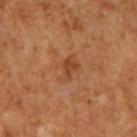Imaged during a routine full-body skin examination; the lesion was not biopsied and no histopathology is available. A male patient in their mid-60s. Imaged with cross-polarized lighting. A 15 mm crop from a total-body photograph taken for skin-cancer surveillance.